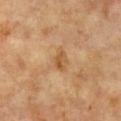Q: Was a biopsy performed?
A: total-body-photography surveillance lesion; no biopsy
Q: How was the tile lit?
A: cross-polarized
Q: What is the lesion's diameter?
A: ≈3 mm
Q: What are the patient's age and sex?
A: female, aged 73–77
Q: What is the anatomic site?
A: the upper back
Q: What is the imaging modality?
A: total-body-photography crop, ~15 mm field of view